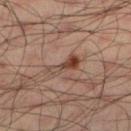<record>
<biopsy_status>not biopsied; imaged during a skin examination</biopsy_status>
<lesion_size>
  <long_diameter_mm_approx>4.0</long_diameter_mm_approx>
</lesion_size>
<lighting>cross-polarized</lighting>
<image>
  <source>total-body photography crop</source>
  <field_of_view_mm>15</field_of_view_mm>
</image>
<site>left lower leg</site>
<patient>
  <sex>male</sex>
  <age_approx>35</age_approx>
</patient>
</record>